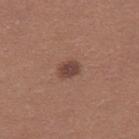Q: Patient demographics?
A: female, aged around 20
Q: How was this image acquired?
A: total-body-photography crop, ~15 mm field of view
Q: What is the anatomic site?
A: the right thigh
Q: What did automated image analysis measure?
A: an eccentricity of roughly 0.65 and a symmetry-axis asymmetry near 0.25
Q: Lesion size?
A: ~2.5 mm (longest diameter)